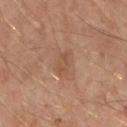Notes:
* notes — total-body-photography surveillance lesion; no biopsy
* lesion diameter — about 3 mm
* image — 15 mm crop, total-body photography
* anatomic site — the right forearm
* automated lesion analysis — a lesion color around L≈50 a*≈19 b*≈31 in CIELAB and about 6 CIELAB-L* units darker than the surrounding skin
* subject — male, aged 43–47